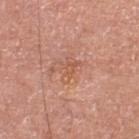Case summary:
* follow-up: total-body-photography surveillance lesion; no biopsy
* image source: 15 mm crop, total-body photography
* site: the left lower leg
* patient: male, in their 80s